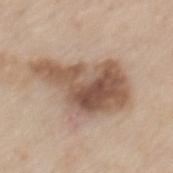Recorded during total-body skin imaging; not selected for excision or biopsy.
Cropped from a total-body skin-imaging series; the visible field is about 15 mm.
A male subject, in their mid-60s.
On the back.